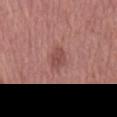Recorded during total-body skin imaging; not selected for excision or biopsy.
Located on the mid back.
An algorithmic analysis of the crop reported a footprint of about 4 mm², an eccentricity of roughly 0.7, and a symmetry-axis asymmetry near 0.25. It also reported a color-variation rating of about 2/10 and peripheral color asymmetry of about 0.5. The software also gave a nevus-likeness score of about 30/100 and a lesion-detection confidence of about 100/100.
A region of skin cropped from a whole-body photographic capture, roughly 15 mm wide.
The tile uses white-light illumination.
The subject is a male aged around 65.
Longest diameter approximately 2.5 mm.